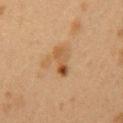Findings:
* biopsy status · catalogued during a skin exam; not biopsied
* image · 15 mm crop, total-body photography
* diameter · ~3.5 mm (longest diameter)
* anatomic site · the arm
* lighting · cross-polarized illumination
* patient · female, roughly 40 years of age